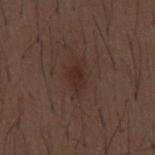| key | value |
|---|---|
| biopsy status | imaged on a skin check; not biopsied |
| anatomic site | the mid back |
| patient | male, aged 48–52 |
| lesion diameter | about 3.5 mm |
| illumination | white-light |
| TBP lesion metrics | an area of roughly 5.5 mm², an outline eccentricity of about 0.85 (0 = round, 1 = elongated), and two-axis asymmetry of about 0.4; an average lesion color of about L≈28 a*≈16 b*≈22 (CIELAB), roughly 6 lightness units darker than nearby skin, and a lesion-to-skin contrast of about 6.5 (normalized; higher = more distinct); an automated nevus-likeness rating near 20 out of 100 |
| imaging modality | ~15 mm tile from a whole-body skin photo |